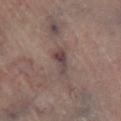Imaged during a routine full-body skin examination; the lesion was not biopsied and no histopathology is available. Located on the right lower leg. This image is a 15 mm lesion crop taken from a total-body photograph.